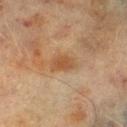The lesion was tiled from a total-body skin photograph and was not biopsied.
A 15 mm crop from a total-body photograph taken for skin-cancer surveillance.
The lesion is located on the right lower leg.
Imaged with cross-polarized lighting.
A male subject aged approximately 70.
Longest diameter approximately 3.5 mm.
An algorithmic analysis of the crop reported an outline eccentricity of about 0.8 (0 = round, 1 = elongated) and a symmetry-axis asymmetry near 0.25.